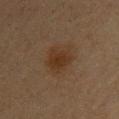follow-up — total-body-photography surveillance lesion; no biopsy
lighting — cross-polarized illumination
subject — female, aged around 45
size — ~4 mm (longest diameter)
location — the upper back
image source — ~15 mm crop, total-body skin-cancer survey
image-analysis metrics — an area of roughly 10 mm² and an outline eccentricity of about 0.45 (0 = round, 1 = elongated); an automated nevus-likeness rating near 75 out of 100 and a lesion-detection confidence of about 100/100